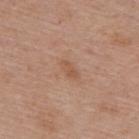Clinical impression:
Part of a total-body skin-imaging series; this lesion was reviewed on a skin check and was not flagged for biopsy.
Image and clinical context:
The lesion-visualizer software estimated a lesion color around L≈54 a*≈21 b*≈31 in CIELAB, about 7 CIELAB-L* units darker than the surrounding skin, and a lesion-to-skin contrast of about 5.5 (normalized; higher = more distinct). And it measured border irregularity of about 2.5 on a 0–10 scale, a within-lesion color-variation index near 0.5/10, and radial color variation of about 0. The recorded lesion diameter is about 2.5 mm. Located on the upper back. Captured under white-light illumination. The subject is a female aged approximately 60. Cropped from a whole-body photographic skin survey; the tile spans about 15 mm.